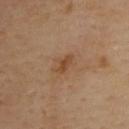Impression:
Recorded during total-body skin imaging; not selected for excision or biopsy.
Background:
A 15 mm crop from a total-body photograph taken for skin-cancer surveillance. The lesion is located on the upper back. Automated image analysis of the tile measured a shape eccentricity near 0.85. The recorded lesion diameter is about 3 mm. This is a cross-polarized tile. A male patient, in their mid- to late 50s.